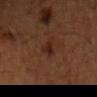<lesion>
<biopsy_status>not biopsied; imaged during a skin examination</biopsy_status>
<lesion_size>
  <long_diameter_mm_approx>2.5</long_diameter_mm_approx>
</lesion_size>
<automated_metrics>
  <eccentricity>0.8</eccentricity>
  <shape_asymmetry>0.35</shape_asymmetry>
  <cielab_L>23</cielab_L>
  <cielab_a>20</cielab_a>
  <cielab_b>24</cielab_b>
  <vs_skin_darker_L>7.0</vs_skin_darker_L>
  <color_variation_0_10>2.0</color_variation_0_10>
  <peripheral_color_asymmetry>1.0</peripheral_color_asymmetry>
  <nevus_likeness_0_100>60</nevus_likeness_0_100>
</automated_metrics>
<patient>
  <sex>male</sex>
  <age_approx>45</age_approx>
</patient>
<site>front of the torso</site>
<image>
  <source>total-body photography crop</source>
  <field_of_view_mm>15</field_of_view_mm>
</image>
<lighting>cross-polarized</lighting>
</lesion>